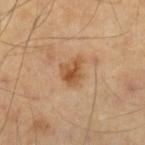{"biopsy_status": "not biopsied; imaged during a skin examination", "lesion_size": {"long_diameter_mm_approx": 3.0}, "lighting": "cross-polarized", "site": "arm", "patient": {"sex": "male", "age_approx": 55}, "image": {"source": "total-body photography crop", "field_of_view_mm": 15}}